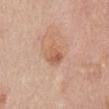Clinical impression:
Part of a total-body skin-imaging series; this lesion was reviewed on a skin check and was not flagged for biopsy.
Background:
From the front of the torso. The lesion's longest dimension is about 3 mm. Automated tile analysis of the lesion measured an average lesion color of about L≈60 a*≈22 b*≈32 (CIELAB), a lesion–skin lightness drop of about 8, and a lesion-to-skin contrast of about 5.5 (normalized; higher = more distinct). The analysis additionally found a border-irregularity rating of about 1.5/10 and internal color variation of about 5 on a 0–10 scale. And it measured an automated nevus-likeness rating near 5 out of 100 and a lesion-detection confidence of about 100/100. A female subject, about 65 years old. The tile uses white-light illumination. A 15 mm close-up tile from a total-body photography series done for melanoma screening.